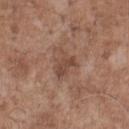Q: Was this lesion biopsied?
A: no biopsy performed (imaged during a skin exam)
Q: What kind of image is this?
A: total-body-photography crop, ~15 mm field of view
Q: Who is the patient?
A: male, roughly 70 years of age
Q: What is the anatomic site?
A: the chest